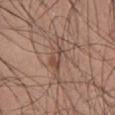Assessment: The lesion was tiled from a total-body skin photograph and was not biopsied. Context: A roughly 15 mm field-of-view crop from a total-body skin photograph. This is a white-light tile. A male subject, aged approximately 30. On the chest. Approximately 5.5 mm at its widest. The lesion-visualizer software estimated an area of roughly 6.5 mm², a shape eccentricity near 0.95, and two-axis asymmetry of about 0.5. The software also gave an average lesion color of about L≈49 a*≈18 b*≈25 (CIELAB) and a lesion-to-skin contrast of about 5.5 (normalized; higher = more distinct). It also reported border irregularity of about 6.5 on a 0–10 scale, internal color variation of about 3 on a 0–10 scale, and a peripheral color-asymmetry measure near 0.5. It also reported a nevus-likeness score of about 0/100 and lesion-presence confidence of about 65/100.